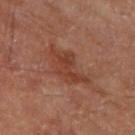The lesion was tiled from a total-body skin photograph and was not biopsied. The recorded lesion diameter is about 6 mm. The tile uses cross-polarized illumination. A 15 mm close-up extracted from a 3D total-body photography capture. A male subject, approximately 70 years of age. The lesion is on the left thigh.